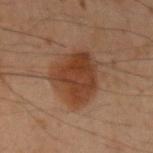Case summary:
* image — ~15 mm tile from a whole-body skin photo
* lesion size — about 5 mm
* tile lighting — cross-polarized
* location — the left upper arm
* subject — male, in their 50s
* automated metrics — a footprint of about 21 mm², an outline eccentricity of about 0.35 (0 = round, 1 = elongated), and two-axis asymmetry of about 0.25; roughly 9 lightness units darker than nearby skin